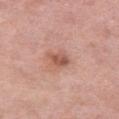The lesion is on the left thigh. Measured at roughly 2.5 mm in maximum diameter. Cropped from a whole-body photographic skin survey; the tile spans about 15 mm. A female patient, aged around 40. Imaged with white-light lighting.